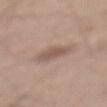Findings:
* follow-up: no biopsy performed (imaged during a skin exam)
* subject: male, aged around 65
* tile lighting: white-light
* image: ~15 mm tile from a whole-body skin photo
* diameter: ~3.5 mm (longest diameter)
* location: the mid back